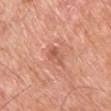biopsy_status: not biopsied; imaged during a skin examination
patient:
  sex: male
  age_approx: 60
lighting: white-light
site: chest
lesion_size:
  long_diameter_mm_approx: 3.0
automated_metrics:
  color_variation_0_10: 4.0
  peripheral_color_asymmetry: 1.5
image:
  source: total-body photography crop
  field_of_view_mm: 15